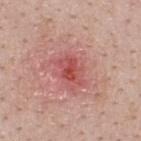- workup — total-body-photography surveillance lesion; no biopsy
- subject — male, roughly 50 years of age
- site — the upper back
- lesion diameter — about 4 mm
- tile lighting — white-light
- image — total-body-photography crop, ~15 mm field of view
- automated lesion analysis — a lesion color around L≈52 a*≈33 b*≈26 in CIELAB, roughly 10 lightness units darker than nearby skin, and a lesion-to-skin contrast of about 7 (normalized; higher = more distinct)